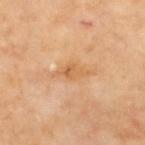Captured during whole-body skin photography for melanoma surveillance; the lesion was not biopsied. The subject is a female in their mid-60s. Automated image analysis of the tile measured a lesion area of about 3.5 mm², a shape eccentricity near 0.85, and two-axis asymmetry of about 0.35. The tile uses cross-polarized illumination. On the upper back. Longest diameter approximately 3.5 mm. A close-up tile cropped from a whole-body skin photograph, about 15 mm across.